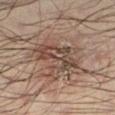Captured under cross-polarized illumination. Cropped from a total-body skin-imaging series; the visible field is about 15 mm. The lesion's longest dimension is about 6.5 mm. The lesion is on the left lower leg. The total-body-photography lesion software estimated an area of roughly 17 mm² and two-axis asymmetry of about 0.4. The analysis additionally found border irregularity of about 7 on a 0–10 scale and a peripheral color-asymmetry measure near 2. A male patient, aged around 45.